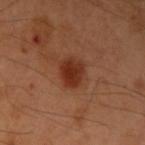No biopsy was performed on this lesion — it was imaged during a full skin examination and was not determined to be concerning. The total-body-photography lesion software estimated an area of roughly 8 mm², an outline eccentricity of about 0.25 (0 = round, 1 = elongated), and a symmetry-axis asymmetry near 0.2. The software also gave an average lesion color of about L≈32 a*≈26 b*≈32 (CIELAB), a lesion–skin lightness drop of about 10, and a normalized lesion–skin contrast near 9.5. The analysis additionally found border irregularity of about 2 on a 0–10 scale and a color-variation rating of about 4/10. It also reported an automated nevus-likeness rating near 100 out of 100 and lesion-presence confidence of about 100/100. A region of skin cropped from a whole-body photographic capture, roughly 15 mm wide. A male patient aged 48–52. Measured at roughly 3 mm in maximum diameter. Located on the left upper arm.